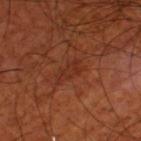Assessment:
No biopsy was performed on this lesion — it was imaged during a full skin examination and was not determined to be concerning.
Acquisition and patient details:
A close-up tile cropped from a whole-body skin photograph, about 15 mm across. The lesion is on the left thigh. Imaged with cross-polarized lighting. About 3.5 mm across. The subject is a male approximately 70 years of age.